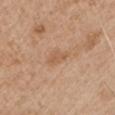The lesion was photographed on a routine skin check and not biopsied; there is no pathology result.
This is a white-light tile.
Located on the left upper arm.
A roughly 15 mm field-of-view crop from a total-body skin photograph.
A male subject, in their mid-60s.